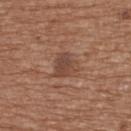Captured during whole-body skin photography for melanoma surveillance; the lesion was not biopsied.
A roughly 15 mm field-of-view crop from a total-body skin photograph.
Captured under white-light illumination.
Measured at roughly 3 mm in maximum diameter.
From the upper back.
The patient is a female in their mid- to late 70s.
Automated tile analysis of the lesion measured a lesion area of about 7.5 mm², an outline eccentricity of about 0.35 (0 = round, 1 = elongated), and a shape-asymmetry score of about 0.3 (0 = symmetric). The software also gave border irregularity of about 3 on a 0–10 scale, a within-lesion color-variation index near 3.5/10, and radial color variation of about 1. It also reported an automated nevus-likeness rating near 10 out of 100 and a detector confidence of about 100 out of 100 that the crop contains a lesion.